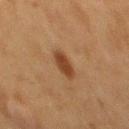Impression:
The lesion was tiled from a total-body skin photograph and was not biopsied.
Acquisition and patient details:
Imaged with cross-polarized lighting. The lesion is located on the back. A male subject aged approximately 75. A 15 mm crop from a total-body photograph taken for skin-cancer surveillance.